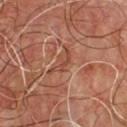Q: Is there a histopathology result?
A: no biopsy performed (imaged during a skin exam)